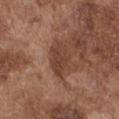Clinical impression: Recorded during total-body skin imaging; not selected for excision or biopsy. Acquisition and patient details: The patient is a male aged 73–77. About 4.5 mm across. The total-body-photography lesion software estimated an area of roughly 8.5 mm², a shape eccentricity near 0.85, and two-axis asymmetry of about 0.2. It also reported an average lesion color of about L≈41 a*≈21 b*≈28 (CIELAB) and a normalized lesion–skin contrast near 8. The software also gave a border-irregularity rating of about 3/10, a within-lesion color-variation index near 2.5/10, and radial color variation of about 1. The software also gave a nevus-likeness score of about 10/100. This is a white-light tile. From the front of the torso. A 15 mm close-up tile from a total-body photography series done for melanoma screening.